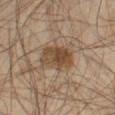Q: Was this lesion biopsied?
A: imaged on a skin check; not biopsied
Q: What did automated image analysis measure?
A: a footprint of about 12 mm², a shape eccentricity near 0.7, and two-axis asymmetry of about 0.15; a border-irregularity index near 2/10, a color-variation rating of about 5/10, and peripheral color asymmetry of about 1.5; an automated nevus-likeness rating near 55 out of 100 and lesion-presence confidence of about 100/100
Q: Who is the patient?
A: male, approximately 55 years of age
Q: What is the anatomic site?
A: the right thigh
Q: How was this image acquired?
A: total-body-photography crop, ~15 mm field of view
Q: How large is the lesion?
A: about 4.5 mm